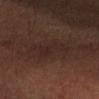workup = total-body-photography surveillance lesion; no biopsy
patient = male, aged 33 to 37
illumination = cross-polarized illumination
lesion diameter = about 4.5 mm
body site = the left forearm
imaging modality = ~15 mm tile from a whole-body skin photo